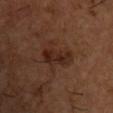Clinical impression:
The lesion was tiled from a total-body skin photograph and was not biopsied.
Background:
A male patient approximately 55 years of age. Located on the chest. Cropped from a total-body skin-imaging series; the visible field is about 15 mm.